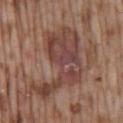{
  "biopsy_status": "not biopsied; imaged during a skin examination",
  "site": "mid back",
  "patient": {
    "sex": "male",
    "age_approx": 75
  },
  "image": {
    "source": "total-body photography crop",
    "field_of_view_mm": 15
  }
}